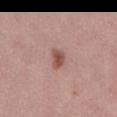Part of a total-body skin-imaging series; this lesion was reviewed on a skin check and was not flagged for biopsy. From the lower back. A close-up tile cropped from a whole-body skin photograph, about 15 mm across. The tile uses white-light illumination. The patient is a female approximately 55 years of age. The lesion-visualizer software estimated a lesion color around L≈52 a*≈23 b*≈25 in CIELAB and a normalized border contrast of about 8.5. The software also gave a nevus-likeness score of about 85/100 and a detector confidence of about 100 out of 100 that the crop contains a lesion. Approximately 2.5 mm at its widest.